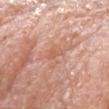<record>
<site>head or neck</site>
<lighting>white-light</lighting>
<image>
  <source>total-body photography crop</source>
  <field_of_view_mm>15</field_of_view_mm>
</image>
<lesion_size>
  <long_diameter_mm_approx>2.5</long_diameter_mm_approx>
</lesion_size>
<patient>
  <sex>male</sex>
  <age_approx>75</age_approx>
</patient>
</record>